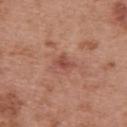Q: Is there a histopathology result?
A: no biopsy performed (imaged during a skin exam)
Q: How was this image acquired?
A: ~15 mm tile from a whole-body skin photo
Q: Where on the body is the lesion?
A: the back
Q: What are the patient's age and sex?
A: female, aged approximately 40